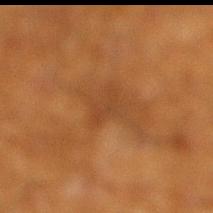The lesion is on the right lower leg. An algorithmic analysis of the crop reported an area of roughly 4.5 mm² and a shape eccentricity near 0.75. The analysis additionally found a mean CIELAB color near L≈36 a*≈20 b*≈33, roughly 5 lightness units darker than nearby skin, and a normalized lesion–skin contrast near 4.5. A male subject aged approximately 60. A roughly 15 mm field-of-view crop from a total-body skin photograph. Imaged with cross-polarized lighting.